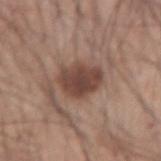{"biopsy_status": "not biopsied; imaged during a skin examination", "image": {"source": "total-body photography crop", "field_of_view_mm": 15}, "patient": {"sex": "male", "age_approx": 45}, "site": "right forearm"}